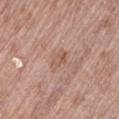Impression:
This lesion was catalogued during total-body skin photography and was not selected for biopsy.
Context:
The lesion-visualizer software estimated a footprint of about 3.5 mm², a shape eccentricity near 0.8, and a symmetry-axis asymmetry near 0.3. A lesion tile, about 15 mm wide, cut from a 3D total-body photograph. The tile uses white-light illumination. The lesion is located on the left thigh. Measured at roughly 2.5 mm in maximum diameter. The patient is a male aged 68–72.